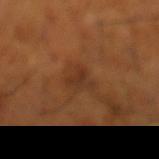<tbp_lesion>
<biopsy_status>not biopsied; imaged during a skin examination</biopsy_status>
<patient>
  <sex>male</sex>
  <age_approx>65</age_approx>
</patient>
<lesion_size>
  <long_diameter_mm_approx>3.5</long_diameter_mm_approx>
</lesion_size>
<image>
  <source>total-body photography crop</source>
  <field_of_view_mm>15</field_of_view_mm>
</image>
<site>left lower leg</site>
</tbp_lesion>